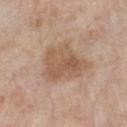The lesion was tiled from a total-body skin photograph and was not biopsied.
Located on the chest.
Approximately 5.5 mm at its widest.
The subject is a male aged around 80.
The tile uses white-light illumination.
A lesion tile, about 15 mm wide, cut from a 3D total-body photograph.
Automated image analysis of the tile measured border irregularity of about 3.5 on a 0–10 scale and radial color variation of about 1.5. The software also gave an automated nevus-likeness rating near 15 out of 100 and lesion-presence confidence of about 100/100.